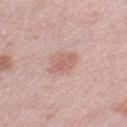notes: catalogued during a skin exam; not biopsied
lighting: white-light illumination
automated metrics: an outline eccentricity of about 0.8 (0 = round, 1 = elongated); a border-irregularity rating of about 2.5/10 and peripheral color asymmetry of about 1
image: ~15 mm crop, total-body skin-cancer survey
location: the leg
size: about 3.5 mm
patient: female, roughly 40 years of age